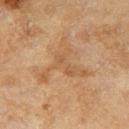Notes:
- follow-up: imaged on a skin check; not biopsied
- site: the leg
- automated lesion analysis: a border-irregularity rating of about 10/10, a color-variation rating of about 3/10, and peripheral color asymmetry of about 0.5; a nevus-likeness score of about 0/100 and a lesion-detection confidence of about 85/100
- acquisition: ~15 mm crop, total-body skin-cancer survey
- patient: female, roughly 60 years of age
- lighting: cross-polarized